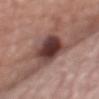The lesion was photographed on a routine skin check and not biopsied; there is no pathology result. Automated image analysis of the tile measured an area of roughly 17 mm² and an eccentricity of roughly 0.95. And it measured a lesion color around L≈40 a*≈20 b*≈21 in CIELAB, about 18 CIELAB-L* units darker than the surrounding skin, and a normalized lesion–skin contrast near 13. On the mid back. A male subject, aged 73 to 77. The lesion's longest dimension is about 8.5 mm. A 15 mm crop from a total-body photograph taken for skin-cancer surveillance.